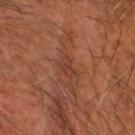A male subject aged 63–67.
A 15 mm crop from a total-body photograph taken for skin-cancer surveillance.
This is a cross-polarized tile.
Automated tile analysis of the lesion measured an area of roughly 3 mm², an outline eccentricity of about 0.8 (0 = round, 1 = elongated), and a shape-asymmetry score of about 0.35 (0 = symmetric).